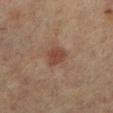Assessment:
No biopsy was performed on this lesion — it was imaged during a full skin examination and was not determined to be concerning.
Clinical summary:
Captured under cross-polarized illumination. A female subject roughly 65 years of age. The lesion is located on the right leg. A 15 mm close-up tile from a total-body photography series done for melanoma screening. Measured at roughly 3 mm in maximum diameter.